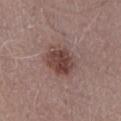A 15 mm crop from a total-body photograph taken for skin-cancer surveillance. A male subject, in their mid-70s. From the abdomen. This is a white-light tile. About 4 mm across. Automated tile analysis of the lesion measured a nevus-likeness score of about 80/100 and lesion-presence confidence of about 100/100.